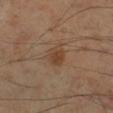| field | value |
|---|---|
| patient | male, about 55 years old |
| automated metrics | a lesion color around L≈42 a*≈17 b*≈30 in CIELAB, about 7 CIELAB-L* units darker than the surrounding skin, and a lesion-to-skin contrast of about 6.5 (normalized; higher = more distinct); border irregularity of about 2 on a 0–10 scale, internal color variation of about 3 on a 0–10 scale, and a peripheral color-asymmetry measure near 1; a nevus-likeness score of about 70/100 and a detector confidence of about 100 out of 100 that the crop contains a lesion |
| lesion diameter | ≈3 mm |
| illumination | cross-polarized |
| acquisition | 15 mm crop, total-body photography |
| site | the right lower leg |